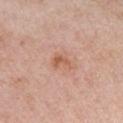Cropped from a total-body skin-imaging series; the visible field is about 15 mm.
A female subject aged around 65.
The lesion is located on the left upper arm.